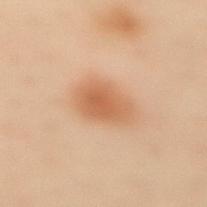{"lighting": "cross-polarized", "automated_metrics": {"area_mm2_approx": 11.0, "shape_asymmetry": 0.2, "vs_skin_darker_L": 9.0, "vs_skin_contrast_norm": 7.0, "color_variation_0_10": 2.5, "peripheral_color_asymmetry": 0.5, "nevus_likeness_0_100": 100, "lesion_detection_confidence_0_100": 100}, "image": {"source": "total-body photography crop", "field_of_view_mm": 15}, "lesion_size": {"long_diameter_mm_approx": 4.0}, "site": "mid back", "patient": {"sex": "female", "age_approx": 60}}